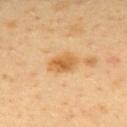The lesion is located on the back. Automated tile analysis of the lesion measured a mean CIELAB color near L≈63 a*≈22 b*≈45, about 11 CIELAB-L* units darker than the surrounding skin, and a normalized border contrast of about 8. This is a cross-polarized tile. A region of skin cropped from a whole-body photographic capture, roughly 15 mm wide. A male patient, aged 38–42.